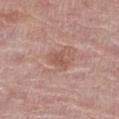lighting=white-light illumination
acquisition=15 mm crop, total-body photography
site=the right thigh
subject=female, aged approximately 65
image-analysis metrics=an area of roughly 3.5 mm² and a symmetry-axis asymmetry near 0.35; a nevus-likeness score of about 0/100 and a lesion-detection confidence of about 100/100